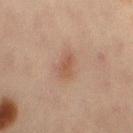workup: total-body-photography surveillance lesion; no biopsy
subject: female, aged 43 to 47
location: the right thigh
image source: total-body-photography crop, ~15 mm field of view